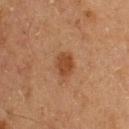This lesion was catalogued during total-body skin photography and was not selected for biopsy.